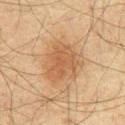Assessment:
Part of a total-body skin-imaging series; this lesion was reviewed on a skin check and was not flagged for biopsy.
Clinical summary:
A male subject, in their mid- to late 60s. The lesion-visualizer software estimated a footprint of about 17 mm², an eccentricity of roughly 0.55, and a symmetry-axis asymmetry near 0.25. And it measured an average lesion color of about L≈48 a*≈17 b*≈32 (CIELAB), roughly 8 lightness units darker than nearby skin, and a lesion-to-skin contrast of about 6.5 (normalized; higher = more distinct). The analysis additionally found a border-irregularity rating of about 3/10 and peripheral color asymmetry of about 1. This is a cross-polarized tile. A 15 mm close-up tile from a total-body photography series done for melanoma screening. The recorded lesion diameter is about 5 mm. On the abdomen.